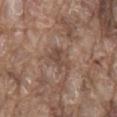The lesion was tiled from a total-body skin photograph and was not biopsied.
An algorithmic analysis of the crop reported a mean CIELAB color near L≈46 a*≈17 b*≈25, roughly 8 lightness units darker than nearby skin, and a normalized lesion–skin contrast near 6.5.
The lesion is located on the mid back.
A 15 mm close-up extracted from a 3D total-body photography capture.
A male subject, in their 80s.
Approximately 3 mm at its widest.